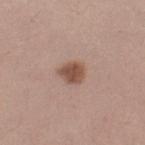follow-up = imaged on a skin check; not biopsied
image source = ~15 mm crop, total-body skin-cancer survey
subject = female, approximately 25 years of age
location = the right lower leg